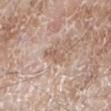No biopsy was performed on this lesion — it was imaged during a full skin examination and was not determined to be concerning.
A region of skin cropped from a whole-body photographic capture, roughly 15 mm wide.
On the left lower leg.
The subject is a male roughly 60 years of age.
This is a white-light tile.
Longest diameter approximately 3 mm.